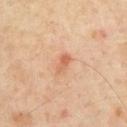Assessment:
The lesion was tiled from a total-body skin photograph and was not biopsied.
Image and clinical context:
On the front of the torso. A male subject, about 70 years old. An algorithmic analysis of the crop reported a lesion area of about 3 mm² and an outline eccentricity of about 0.85 (0 = round, 1 = elongated). The software also gave roughly 9 lightness units darker than nearby skin and a lesion-to-skin contrast of about 6 (normalized; higher = more distinct). It also reported an automated nevus-likeness rating near 10 out of 100 and a lesion-detection confidence of about 100/100. A roughly 15 mm field-of-view crop from a total-body skin photograph. This is a cross-polarized tile.